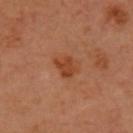workup: catalogued during a skin exam; not biopsied
image source: total-body-photography crop, ~15 mm field of view
anatomic site: the head or neck
patient: female, aged 58–62
automated lesion analysis: an area of roughly 5.5 mm²; an automated nevus-likeness rating near 65 out of 100 and lesion-presence confidence of about 100/100
size: ≈3 mm
tile lighting: cross-polarized